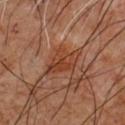Q: Was a biopsy performed?
A: catalogued during a skin exam; not biopsied
Q: Patient demographics?
A: male, approximately 60 years of age
Q: How was this image acquired?
A: ~15 mm crop, total-body skin-cancer survey
Q: Lesion location?
A: the front of the torso
Q: Lesion size?
A: ≈4.5 mm